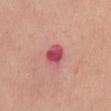Captured during whole-body skin photography for melanoma surveillance; the lesion was not biopsied. The lesion is on the mid back. Longest diameter approximately 3 mm. A male patient approximately 30 years of age. Imaged with white-light lighting. A roughly 15 mm field-of-view crop from a total-body skin photograph. The lesion-visualizer software estimated a lesion area of about 6 mm² and a shape eccentricity near 0.55. The software also gave border irregularity of about 2 on a 0–10 scale and a within-lesion color-variation index near 4/10. The analysis additionally found a nevus-likeness score of about 0/100 and a lesion-detection confidence of about 100/100.